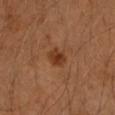Clinical summary:
Longest diameter approximately 2.5 mm. Imaged with cross-polarized lighting. Cropped from a total-body skin-imaging series; the visible field is about 15 mm. Located on the left forearm. A male subject roughly 40 years of age.